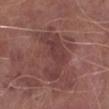Part of a total-body skin-imaging series; this lesion was reviewed on a skin check and was not flagged for biopsy. A close-up tile cropped from a whole-body skin photograph, about 15 mm across. The patient is a male aged around 65. From the leg.